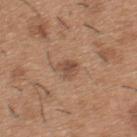On the upper back. Cropped from a total-body skin-imaging series; the visible field is about 15 mm. A male subject aged 38–42.